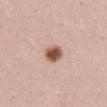Findings:
- follow-up: catalogued during a skin exam; not biopsied
- patient: female, in their mid-40s
- acquisition: ~15 mm crop, total-body skin-cancer survey
- anatomic site: the abdomen
- TBP lesion metrics: a lesion color around L≈55 a*≈22 b*≈28 in CIELAB and about 17 CIELAB-L* units darker than the surrounding skin; border irregularity of about 1.5 on a 0–10 scale, internal color variation of about 5 on a 0–10 scale, and a peripheral color-asymmetry measure near 1.5; a classifier nevus-likeness of about 100/100 and a lesion-detection confidence of about 100/100
- lesion size: ~2.5 mm (longest diameter)
- tile lighting: white-light